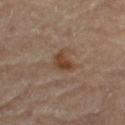biopsy status: no biopsy performed (imaged during a skin exam) | acquisition: total-body-photography crop, ~15 mm field of view | automated lesion analysis: an average lesion color of about L≈40 a*≈17 b*≈28 (CIELAB), roughly 9 lightness units darker than nearby skin, and a normalized lesion–skin contrast near 8.5; border irregularity of about 3 on a 0–10 scale, a within-lesion color-variation index near 2.5/10, and a peripheral color-asymmetry measure near 1; an automated nevus-likeness rating near 60 out of 100 and lesion-presence confidence of about 100/100 | location: the right thigh | lesion diameter: about 3 mm | patient: male, in their mid-80s or older | tile lighting: cross-polarized.